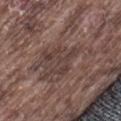Case summary:
* notes: catalogued during a skin exam; not biopsied
* image source: ~15 mm tile from a whole-body skin photo
* location: the leg
* tile lighting: white-light
* size: ≈4 mm
* subject: male, in their mid-70s